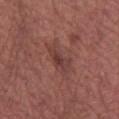| field | value |
|---|---|
| workup | catalogued during a skin exam; not biopsied |
| imaging modality | 15 mm crop, total-body photography |
| subject | male, aged around 55 |
| illumination | white-light |
| body site | the left lower leg |